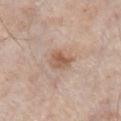notes=no biopsy performed (imaged during a skin exam) | subject=male, roughly 80 years of age | size=~3.5 mm (longest diameter) | body site=the chest | image-analysis metrics=an area of roughly 6.5 mm² and two-axis asymmetry of about 0.2; a nevus-likeness score of about 65/100 and lesion-presence confidence of about 100/100 | acquisition=~15 mm tile from a whole-body skin photo | tile lighting=white-light.